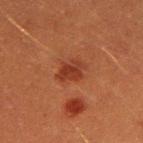The lesion was tiled from a total-body skin photograph and was not biopsied.